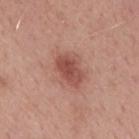The lesion was photographed on a routine skin check and not biopsied; there is no pathology result. The lesion is on the mid back. A 15 mm close-up extracted from a 3D total-body photography capture. The patient is a male aged approximately 50. Approximately 4 mm at its widest.